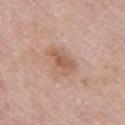Clinical impression:
Part of a total-body skin-imaging series; this lesion was reviewed on a skin check and was not flagged for biopsy.
Clinical summary:
About 4 mm across. The subject is a female aged 28 to 32. The total-body-photography lesion software estimated a lesion area of about 8 mm² and two-axis asymmetry of about 0.35. The software also gave a border-irregularity rating of about 3/10, a color-variation rating of about 4.5/10, and a peripheral color-asymmetry measure near 1.5. This is a white-light tile. The lesion is on the arm. Cropped from a whole-body photographic skin survey; the tile spans about 15 mm.